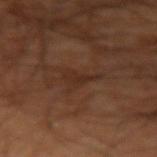notes: imaged on a skin check; not biopsied
patient: male, approximately 60 years of age
site: the left arm
automated metrics: a footprint of about 4.5 mm²; roughly 5 lightness units darker than nearby skin and a normalized lesion–skin contrast near 5.5
tile lighting: cross-polarized illumination
acquisition: 15 mm crop, total-body photography